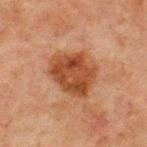<record>
<biopsy_status>not biopsied; imaged during a skin examination</biopsy_status>
<patient>
  <sex>male</sex>
  <age_approx>65</age_approx>
</patient>
<lighting>cross-polarized</lighting>
<automated_metrics>
  <cielab_L>38</cielab_L>
  <cielab_a>22</cielab_a>
  <cielab_b>31</cielab_b>
  <vs_skin_contrast_norm>9.5</vs_skin_contrast_norm>
  <border_irregularity_0_10>2.5</border_irregularity_0_10>
  <color_variation_0_10>5.0</color_variation_0_10>
  <peripheral_color_asymmetry>1.5</peripheral_color_asymmetry>
</automated_metrics>
<image>
  <source>total-body photography crop</source>
  <field_of_view_mm>15</field_of_view_mm>
</image>
<site>front of the torso</site>
</record>